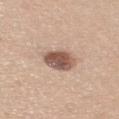The lesion was photographed on a routine skin check and not biopsied; there is no pathology result. Located on the upper back. Longest diameter approximately 3.5 mm. A female patient roughly 30 years of age. A region of skin cropped from a whole-body photographic capture, roughly 15 mm wide. Captured under white-light illumination.